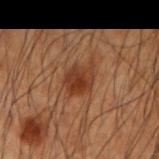Assessment:
Imaged during a routine full-body skin examination; the lesion was not biopsied and no histopathology is available.
Clinical summary:
The tile uses cross-polarized illumination. A 15 mm close-up extracted from a 3D total-body photography capture. Located on the left forearm. Automated tile analysis of the lesion measured a footprint of about 8 mm², an eccentricity of roughly 0.5, and two-axis asymmetry of about 0.2. The analysis additionally found border irregularity of about 2 on a 0–10 scale, a within-lesion color-variation index near 4/10, and radial color variation of about 1.5. The analysis additionally found a detector confidence of about 100 out of 100 that the crop contains a lesion. Measured at roughly 3.5 mm in maximum diameter. A male patient, aged 48–52.